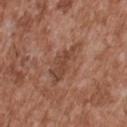biopsy status: imaged on a skin check; not biopsied | size: ≈6 mm | subject: male, in their mid-40s | location: the upper back | automated metrics: a mean CIELAB color near L≈45 a*≈22 b*≈29, about 8 CIELAB-L* units darker than the surrounding skin, and a lesion-to-skin contrast of about 6.5 (normalized; higher = more distinct) | acquisition: ~15 mm crop, total-body skin-cancer survey | tile lighting: white-light illumination.